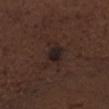Acquisition and patient details:
Measured at roughly 4.5 mm in maximum diameter. The patient is a male about 70 years old. A roughly 15 mm field-of-view crop from a total-body skin photograph. Captured under white-light illumination. The lesion is located on the head or neck.